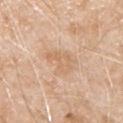{
  "biopsy_status": "not biopsied; imaged during a skin examination",
  "automated_metrics": {
    "area_mm2_approx": 9.0,
    "eccentricity": 0.75,
    "shape_asymmetry": 0.4,
    "cielab_L": 66,
    "cielab_a": 18,
    "cielab_b": 34,
    "vs_skin_darker_L": 6.0,
    "vs_skin_contrast_norm": 4.5,
    "lesion_detection_confidence_0_100": 100
  },
  "patient": {
    "sex": "male",
    "age_approx": 80
  },
  "site": "chest",
  "image": {
    "source": "total-body photography crop",
    "field_of_view_mm": 15
  }
}